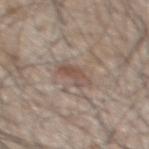Clinical impression: No biopsy was performed on this lesion — it was imaged during a full skin examination and was not determined to be concerning. Acquisition and patient details: The tile uses white-light illumination. A region of skin cropped from a whole-body photographic capture, roughly 15 mm wide. Approximately 3 mm at its widest. Automated tile analysis of the lesion measured about 8 CIELAB-L* units darker than the surrounding skin and a lesion-to-skin contrast of about 6.5 (normalized; higher = more distinct). The software also gave a nevus-likeness score of about 5/100. The patient is a male in their mid- to late 40s. Located on the mid back.